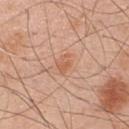{
  "biopsy_status": "not biopsied; imaged during a skin examination",
  "automated_metrics": {
    "area_mm2_approx": 4.0,
    "shape_asymmetry": 0.2,
    "cielab_L": 60,
    "cielab_a": 23,
    "cielab_b": 34
  },
  "patient": {
    "sex": "male",
    "age_approx": 50
  },
  "image": {
    "source": "total-body photography crop",
    "field_of_view_mm": 15
  },
  "lighting": "white-light",
  "lesion_size": {
    "long_diameter_mm_approx": 2.5
  },
  "site": "chest"
}